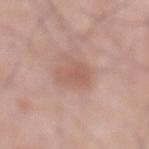This lesion was catalogued during total-body skin photography and was not selected for biopsy.
The tile uses white-light illumination.
A 15 mm close-up tile from a total-body photography series done for melanoma screening.
The recorded lesion diameter is about 3.5 mm.
The subject is a male about 70 years old.
On the abdomen.